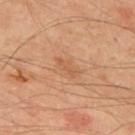The patient is a male aged 63–67. A 15 mm close-up tile from a total-body photography series done for melanoma screening. Longest diameter approximately 3.5 mm. The total-body-photography lesion software estimated a lesion area of about 4 mm², an outline eccentricity of about 0.9 (0 = round, 1 = elongated), and a symmetry-axis asymmetry near 0.35. The software also gave a mean CIELAB color near L≈56 a*≈22 b*≈35 and about 6 CIELAB-L* units darker than the surrounding skin. The software also gave a border-irregularity rating of about 3.5/10 and a peripheral color-asymmetry measure near 1. Imaged with cross-polarized lighting. On the upper back.